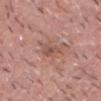Clinical impression:
Imaged during a routine full-body skin examination; the lesion was not biopsied and no histopathology is available.
Image and clinical context:
The subject is a male in their 40s. A 15 mm crop from a total-body photograph taken for skin-cancer surveillance. The lesion is on the head or neck. The lesion-visualizer software estimated a lesion color around L≈52 a*≈21 b*≈25 in CIELAB, a lesion–skin lightness drop of about 8, and a normalized lesion–skin contrast near 6. The software also gave a color-variation rating of about 2.5/10 and radial color variation of about 0.5. Captured under white-light illumination.